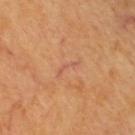The lesion was tiled from a total-body skin photograph and was not biopsied. The lesion is on the upper back. A close-up tile cropped from a whole-body skin photograph, about 15 mm across. The patient is aged around 60. The tile uses cross-polarized illumination.